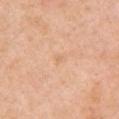Clinical impression: No biopsy was performed on this lesion — it was imaged during a full skin examination and was not determined to be concerning. Image and clinical context: A roughly 15 mm field-of-view crop from a total-body skin photograph. An algorithmic analysis of the crop reported an outline eccentricity of about 0.65 (0 = round, 1 = elongated) and two-axis asymmetry of about 0.5. It also reported a lesion color around L≈69 a*≈20 b*≈37 in CIELAB, a lesion–skin lightness drop of about 5, and a normalized border contrast of about 4. A female patient aged approximately 55. The lesion's longest dimension is about 1 mm. The lesion is on the left upper arm.